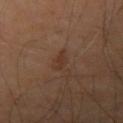notes: no biopsy performed (imaged during a skin exam)
illumination: cross-polarized illumination
patient: male, roughly 70 years of age
site: the right forearm
image source: ~15 mm crop, total-body skin-cancer survey